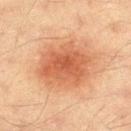Recorded during total-body skin imaging; not selected for excision or biopsy.
The lesion is on the left thigh.
The patient is a male aged 38 to 42.
This is a cross-polarized tile.
A 15 mm close-up extracted from a 3D total-body photography capture.